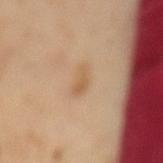Case summary:
• biopsy status · catalogued during a skin exam; not biopsied
• image source · 15 mm crop, total-body photography
• lesion size · about 3 mm
• automated lesion analysis · a lesion area of about 4.5 mm² and an outline eccentricity of about 0.75 (0 = round, 1 = elongated)
• patient · male, aged around 65
• lighting · cross-polarized illumination